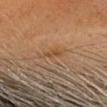Assessment:
Part of a total-body skin-imaging series; this lesion was reviewed on a skin check and was not flagged for biopsy.
Image and clinical context:
A roughly 15 mm field-of-view crop from a total-body skin photograph. The lesion is located on the head or neck. A female patient aged approximately 65.